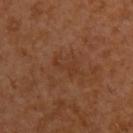Assessment:
Captured during whole-body skin photography for melanoma surveillance; the lesion was not biopsied.
Context:
A male patient approximately 30 years of age. The lesion's longest dimension is about 3.5 mm. The lesion is on the upper back. A roughly 15 mm field-of-view crop from a total-body skin photograph.